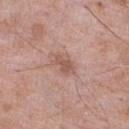Clinical impression: The lesion was tiled from a total-body skin photograph and was not biopsied. Background: A 15 mm close-up extracted from a 3D total-body photography capture. From the right lower leg. Automated image analysis of the tile measured a lesion area of about 4.5 mm², an outline eccentricity of about 0.75 (0 = round, 1 = elongated), and a symmetry-axis asymmetry near 0.3. It also reported border irregularity of about 3.5 on a 0–10 scale, internal color variation of about 2 on a 0–10 scale, and peripheral color asymmetry of about 0.5. The analysis additionally found an automated nevus-likeness rating near 20 out of 100 and lesion-presence confidence of about 100/100. The lesion's longest dimension is about 3 mm. A male patient aged 73–77. The tile uses white-light illumination.